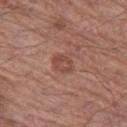The lesion was photographed on a routine skin check and not biopsied; there is no pathology result. Imaged with white-light lighting. Located on the left thigh. The patient is a male approximately 75 years of age. Automated image analysis of the tile measured a mean CIELAB color near L≈47 a*≈23 b*≈26, roughly 8 lightness units darker than nearby skin, and a normalized border contrast of about 6. A 15 mm close-up extracted from a 3D total-body photography capture. The lesion's longest dimension is about 3 mm.